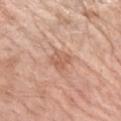lesion size = ~2.5 mm (longest diameter); subject = female, aged 63–67; location = the left forearm; image = 15 mm crop, total-body photography.